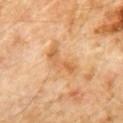Q: Was this lesion biopsied?
A: catalogued during a skin exam; not biopsied
Q: What are the patient's age and sex?
A: male, in their 60s
Q: What did automated image analysis measure?
A: a footprint of about 7.5 mm², a shape eccentricity near 0.9, and two-axis asymmetry of about 0.55; a lesion–skin lightness drop of about 9; a border-irregularity index near 6.5/10, a color-variation rating of about 4.5/10, and a peripheral color-asymmetry measure near 1; a nevus-likeness score of about 0/100 and a lesion-detection confidence of about 100/100
Q: Lesion location?
A: the chest
Q: What is the lesion's diameter?
A: about 5 mm
Q: How was this image acquired?
A: total-body-photography crop, ~15 mm field of view
Q: How was the tile lit?
A: cross-polarized